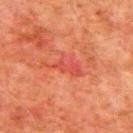<case>
<biopsy_status>not biopsied; imaged during a skin examination</biopsy_status>
<site>upper back</site>
<patient>
  <sex>male</sex>
  <age_approx>80</age_approx>
</patient>
<image>
  <source>total-body photography crop</source>
  <field_of_view_mm>15</field_of_view_mm>
</image>
</case>